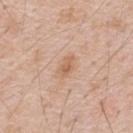The lesion was photographed on a routine skin check and not biopsied; there is no pathology result.
This is a white-light tile.
On the back.
A 15 mm crop from a total-body photograph taken for skin-cancer surveillance.
Longest diameter approximately 3 mm.
A male subject, aged 48 to 52.